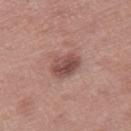Clinical impression:
No biopsy was performed on this lesion — it was imaged during a full skin examination and was not determined to be concerning.